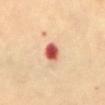This lesion was catalogued during total-body skin photography and was not selected for biopsy. Cropped from a whole-body photographic skin survey; the tile spans about 15 mm. Measured at roughly 2.5 mm in maximum diameter. An algorithmic analysis of the crop reported a footprint of about 5.5 mm², a shape eccentricity near 0.65, and two-axis asymmetry of about 0.2. The analysis additionally found an automated nevus-likeness rating near 0 out of 100 and a lesion-detection confidence of about 100/100. The lesion is on the abdomen. A female patient aged approximately 60.